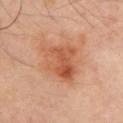| key | value |
|---|---|
| biopsy status | imaged on a skin check; not biopsied |
| image source | 15 mm crop, total-body photography |
| illumination | cross-polarized |
| size | ≈5 mm |
| site | the chest |